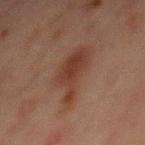Q: What kind of image is this?
A: ~15 mm crop, total-body skin-cancer survey
Q: Where on the body is the lesion?
A: the abdomen
Q: Lesion size?
A: ~7 mm (longest diameter)
Q: Patient demographics?
A: male, aged 63 to 67
Q: Automated lesion metrics?
A: an average lesion color of about L≈30 a*≈17 b*≈23 (CIELAB); a color-variation rating of about 2.5/10 and peripheral color asymmetry of about 0.5